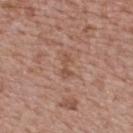{
  "image": {
    "source": "total-body photography crop",
    "field_of_view_mm": 15
  },
  "site": "back",
  "patient": {
    "sex": "female",
    "age_approx": 65
  }
}